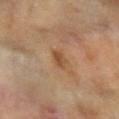Imaged during a routine full-body skin examination; the lesion was not biopsied and no histopathology is available. A female patient, approximately 60 years of age. On the left forearm. About 2.5 mm across. Cropped from a whole-body photographic skin survey; the tile spans about 15 mm. Imaged with cross-polarized lighting.